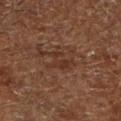notes = total-body-photography surveillance lesion; no biopsy | patient = aged around 65 | lighting = cross-polarized | image = ~15 mm crop, total-body skin-cancer survey | site = the leg | lesion diameter = ~2.5 mm (longest diameter).